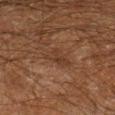The lesion was tiled from a total-body skin photograph and was not biopsied. This is a cross-polarized tile. Longest diameter approximately 3.5 mm. From the right lower leg. The subject is a male aged 58 to 62. A region of skin cropped from a whole-body photographic capture, roughly 15 mm wide.